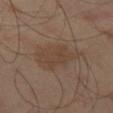The lesion was tiled from a total-body skin photograph and was not biopsied.
The subject is a male aged 43 to 47.
Cropped from a total-body skin-imaging series; the visible field is about 15 mm.
Located on the left thigh.